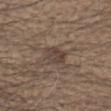Impression:
This lesion was catalogued during total-body skin photography and was not selected for biopsy.
Context:
The lesion is on the head or neck. The patient is a male aged around 70. A 15 mm close-up extracted from a 3D total-body photography capture.